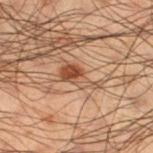– biopsy status · imaged on a skin check; not biopsied
– image source · ~15 mm crop, total-body skin-cancer survey
– site · the left thigh
– diameter · ≈4.5 mm
– patient · male, aged 48 to 52